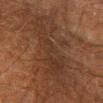Q: Was a biopsy performed?
A: no biopsy performed (imaged during a skin exam)
Q: What lighting was used for the tile?
A: cross-polarized
Q: How large is the lesion?
A: ~9 mm (longest diameter)
Q: What is the anatomic site?
A: the left lower leg
Q: Patient demographics?
A: male, in their 60s
Q: What kind of image is this?
A: ~15 mm tile from a whole-body skin photo